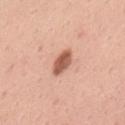| feature | finding |
|---|---|
| automated lesion analysis | an outline eccentricity of about 0.85 (0 = round, 1 = elongated) and a shape-asymmetry score of about 0.25 (0 = symmetric); a mean CIELAB color near L≈58 a*≈25 b*≈31 and a normalized lesion–skin contrast near 9.5; border irregularity of about 2.5 on a 0–10 scale, a color-variation rating of about 2.5/10, and a peripheral color-asymmetry measure near 1 |
| body site | the mid back |
| lesion diameter | ~3.5 mm (longest diameter) |
| imaging modality | ~15 mm tile from a whole-body skin photo |
| subject | male, about 55 years old |